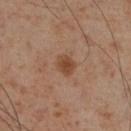Impression:
No biopsy was performed on this lesion — it was imaged during a full skin examination and was not determined to be concerning.
Clinical summary:
The lesion is on the right lower leg. A roughly 15 mm field-of-view crop from a total-body skin photograph. A male subject, in their mid- to late 50s.